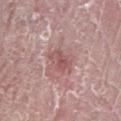Captured during whole-body skin photography for melanoma surveillance; the lesion was not biopsied. This image is a 15 mm lesion crop taken from a total-body photograph. The lesion is on the leg. A male subject, roughly 55 years of age. The tile uses white-light illumination.